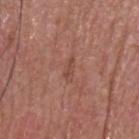Q: Was a biopsy performed?
A: imaged on a skin check; not biopsied
Q: What is the lesion's diameter?
A: ≈3 mm
Q: What is the imaging modality?
A: 15 mm crop, total-body photography
Q: Automated lesion metrics?
A: about 6 CIELAB-L* units darker than the surrounding skin and a lesion-to-skin contrast of about 5 (normalized; higher = more distinct); radial color variation of about 0
Q: What are the patient's age and sex?
A: male, aged approximately 55
Q: Where on the body is the lesion?
A: the head or neck